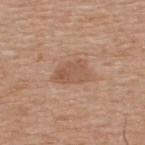Recorded during total-body skin imaging; not selected for excision or biopsy. A male subject, roughly 65 years of age. A lesion tile, about 15 mm wide, cut from a 3D total-body photograph. The lesion is located on the upper back.